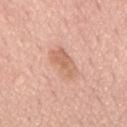Captured during whole-body skin photography for melanoma surveillance; the lesion was not biopsied. About 4.5 mm across. Imaged with white-light lighting. A male subject, in their mid-70s. Automated image analysis of the tile measured a footprint of about 9 mm², an outline eccentricity of about 0.85 (0 = round, 1 = elongated), and a symmetry-axis asymmetry near 0.2. And it measured roughly 9 lightness units darker than nearby skin and a normalized border contrast of about 6. It also reported an automated nevus-likeness rating near 0 out of 100 and a lesion-detection confidence of about 100/100. The lesion is on the back. A 15 mm close-up tile from a total-body photography series done for melanoma screening.